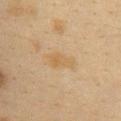Notes:
– follow-up — no biopsy performed (imaged during a skin exam)
– automated lesion analysis — a lesion area of about 5 mm², a shape eccentricity near 0.85, and a symmetry-axis asymmetry near 0.35; border irregularity of about 3.5 on a 0–10 scale, a within-lesion color-variation index near 2/10, and radial color variation of about 0.5
– site — the chest
– image source — ~15 mm tile from a whole-body skin photo
– lesion size — ≈3.5 mm
– subject — female, approximately 40 years of age